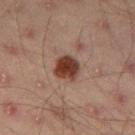workup = catalogued during a skin exam; not biopsied | location = the left thigh | size = ≈3.5 mm | subject = male, aged approximately 45 | automated metrics = a classifier nevus-likeness of about 95/100 and a detector confidence of about 100 out of 100 that the crop contains a lesion | imaging modality = ~15 mm tile from a whole-body skin photo.